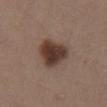A female subject about 40 years old. Located on the left thigh. Automated image analysis of the tile measured an area of roughly 12 mm² and two-axis asymmetry of about 0.3. The software also gave an average lesion color of about L≈37 a*≈16 b*≈23 (CIELAB) and a lesion–skin lightness drop of about 14. The software also gave a border-irregularity rating of about 2.5/10, a within-lesion color-variation index near 4.5/10, and a peripheral color-asymmetry measure near 1.5. Approximately 4.5 mm at its widest. A 15 mm crop from a total-body photograph taken for skin-cancer surveillance. Imaged with white-light lighting.